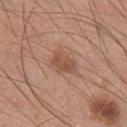Captured during whole-body skin photography for melanoma surveillance; the lesion was not biopsied. A 15 mm close-up extracted from a 3D total-body photography capture. On the chest. Automated tile analysis of the lesion measured a footprint of about 5 mm², an outline eccentricity of about 0.8 (0 = round, 1 = elongated), and two-axis asymmetry of about 0.25. The analysis additionally found a lesion color around L≈51 a*≈22 b*≈30 in CIELAB, about 9 CIELAB-L* units darker than the surrounding skin, and a normalized lesion–skin contrast near 7. A male patient, roughly 25 years of age.